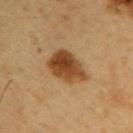Q: Was this lesion biopsied?
A: catalogued during a skin exam; not biopsied
Q: What lighting was used for the tile?
A: cross-polarized illumination
Q: What kind of image is this?
A: total-body-photography crop, ~15 mm field of view
Q: Who is the patient?
A: male, aged around 60
Q: What is the lesion's diameter?
A: about 5 mm
Q: Where on the body is the lesion?
A: the right upper arm